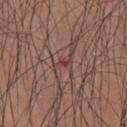Part of a total-body skin-imaging series; this lesion was reviewed on a skin check and was not flagged for biopsy.